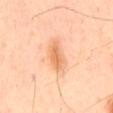Clinical impression: Part of a total-body skin-imaging series; this lesion was reviewed on a skin check and was not flagged for biopsy. Image and clinical context: On the mid back. Automated image analysis of the tile measured a lesion area of about 7.5 mm² and a shape eccentricity near 0.95. And it measured an average lesion color of about L≈71 a*≈24 b*≈40 (CIELAB), roughly 10 lightness units darker than nearby skin, and a normalized border contrast of about 7. The software also gave border irregularity of about 3 on a 0–10 scale and a within-lesion color-variation index near 3/10. The software also gave an automated nevus-likeness rating near 50 out of 100 and lesion-presence confidence of about 100/100. Longest diameter approximately 5 mm. Cropped from a total-body skin-imaging series; the visible field is about 15 mm. Imaged with cross-polarized lighting. The patient is a male about 45 years old.